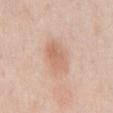Impression:
The lesion was tiled from a total-body skin photograph and was not biopsied.
Background:
A 15 mm crop from a total-body photograph taken for skin-cancer surveillance. The lesion is on the abdomen. A male patient about 60 years old.